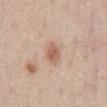Q: Is there a histopathology result?
A: imaged on a skin check; not biopsied
Q: Patient demographics?
A: male, aged approximately 50
Q: What is the anatomic site?
A: the abdomen
Q: What is the imaging modality?
A: ~15 mm crop, total-body skin-cancer survey
Q: What lighting was used for the tile?
A: white-light
Q: What is the lesion's diameter?
A: ~2.5 mm (longest diameter)